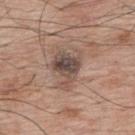The lesion was tiled from a total-body skin photograph and was not biopsied.
The tile uses white-light illumination.
A close-up tile cropped from a whole-body skin photograph, about 15 mm across.
From the upper back.
A male subject, roughly 70 years of age.
The lesion's longest dimension is about 5 mm.